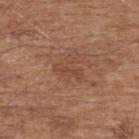Case summary:
• biopsy status — total-body-photography surveillance lesion; no biopsy
• patient — male, approximately 75 years of age
• size — about 3.5 mm
• illumination — white-light illumination
• image-analysis metrics — an average lesion color of about L≈46 a*≈21 b*≈29 (CIELAB), roughly 6 lightness units darker than nearby skin, and a normalized lesion–skin contrast near 4.5
• site — the back
• image — ~15 mm crop, total-body skin-cancer survey